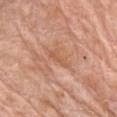Q: Is there a histopathology result?
A: catalogued during a skin exam; not biopsied
Q: What kind of image is this?
A: 15 mm crop, total-body photography
Q: What are the patient's age and sex?
A: male, aged 78 to 82
Q: Where on the body is the lesion?
A: the front of the torso